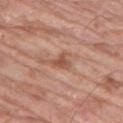Case summary:
* follow-up: total-body-photography surveillance lesion; no biopsy
* patient: male, aged around 80
* image source: ~15 mm tile from a whole-body skin photo
* anatomic site: the left thigh